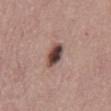{
  "patient": {
    "sex": "male",
    "age_approx": 55
  },
  "lesion_size": {
    "long_diameter_mm_approx": 3.0
  },
  "automated_metrics": {
    "cielab_L": 44,
    "cielab_a": 18,
    "cielab_b": 21,
    "vs_skin_darker_L": 18.0,
    "vs_skin_contrast_norm": 13.0,
    "nevus_likeness_0_100": 95,
    "lesion_detection_confidence_0_100": 100
  },
  "image": {
    "source": "total-body photography crop",
    "field_of_view_mm": 15
  }
}